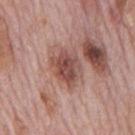The lesion was tiled from a total-body skin photograph and was not biopsied.
A male patient, roughly 75 years of age.
Longest diameter approximately 4.5 mm.
Captured under white-light illumination.
Cropped from a whole-body photographic skin survey; the tile spans about 15 mm.
The lesion is on the mid back.
The total-body-photography lesion software estimated roughly 12 lightness units darker than nearby skin and a normalized border contrast of about 8.5. The analysis additionally found a within-lesion color-variation index near 5.5/10 and radial color variation of about 1.5.